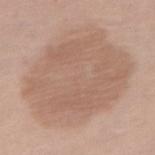Impression: The lesion was photographed on a routine skin check and not biopsied; there is no pathology result. Acquisition and patient details: Cropped from a whole-body photographic skin survey; the tile spans about 15 mm. Located on the right thigh. Measured at roughly 11 mm in maximum diameter. A female patient approximately 55 years of age. This is a white-light tile.